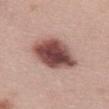biopsy status — no biopsy performed (imaged during a skin exam) | automated metrics — a footprint of about 20 mm², an eccentricity of roughly 0.85, and a symmetry-axis asymmetry near 0.2; a lesion color around L≈48 a*≈22 b*≈22 in CIELAB and a normalized border contrast of about 13.5; a color-variation rating of about 8/10 and radial color variation of about 2.5; a classifier nevus-likeness of about 95/100 and lesion-presence confidence of about 100/100 | body site — the mid back | patient — female, roughly 60 years of age | lighting — white-light illumination | image — ~15 mm crop, total-body skin-cancer survey.